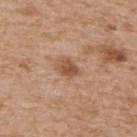| field | value |
|---|---|
| biopsy status | imaged on a skin check; not biopsied |
| body site | the upper back |
| size | about 2.5 mm |
| image-analysis metrics | two-axis asymmetry of about 0.25; a border-irregularity index near 2/10, internal color variation of about 3.5 on a 0–10 scale, and radial color variation of about 1.5; lesion-presence confidence of about 100/100 |
| imaging modality | 15 mm crop, total-body photography |
| subject | male, aged 63–67 |